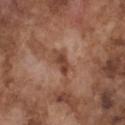Assessment:
This lesion was catalogued during total-body skin photography and was not selected for biopsy.